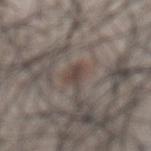biopsy_status: not biopsied; imaged during a skin examination
image:
  source: total-body photography crop
  field_of_view_mm: 15
patient:
  sex: male
  age_approx: 65
site: chest
lesion_size:
  long_diameter_mm_approx: 3.0